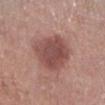biopsy status: no biopsy performed (imaged during a skin exam); patient: female, aged approximately 65; size: ≈5.5 mm; acquisition: 15 mm crop, total-body photography; tile lighting: white-light illumination; anatomic site: the left lower leg.